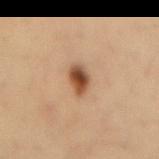Captured during whole-body skin photography for melanoma surveillance; the lesion was not biopsied. A male subject, aged 33–37. A 15 mm close-up tile from a total-body photography series done for melanoma screening. About 3.5 mm across. Automated tile analysis of the lesion measured a footprint of about 5.5 mm² and an eccentricity of roughly 0.75. And it measured an average lesion color of about L≈45 a*≈20 b*≈31 (CIELAB) and a normalized lesion–skin contrast near 11. The software also gave border irregularity of about 2 on a 0–10 scale and a color-variation rating of about 7/10. The lesion is on the lower back.